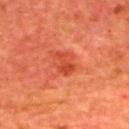Captured during whole-body skin photography for melanoma surveillance; the lesion was not biopsied.
This is a cross-polarized tile.
Cropped from a total-body skin-imaging series; the visible field is about 15 mm.
Approximately 3 mm at its widest.
Automated image analysis of the tile measured a footprint of about 4 mm², an outline eccentricity of about 0.75 (0 = round, 1 = elongated), and a symmetry-axis asymmetry near 0.45. And it measured a lesion color around L≈45 a*≈38 b*≈39 in CIELAB and about 8 CIELAB-L* units darker than the surrounding skin. It also reported border irregularity of about 5 on a 0–10 scale and a within-lesion color-variation index near 1/10. And it measured a nevus-likeness score of about 80/100 and a detector confidence of about 100 out of 100 that the crop contains a lesion.
A male patient in their mid-60s.
From the upper back.